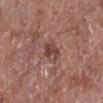Clinical impression:
No biopsy was performed on this lesion — it was imaged during a full skin examination and was not determined to be concerning.
Image and clinical context:
Cropped from a whole-body photographic skin survey; the tile spans about 15 mm. A male subject, in their mid-70s. The lesion is located on the right lower leg.